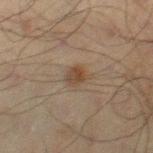The lesion was tiled from a total-body skin photograph and was not biopsied. The total-body-photography lesion software estimated a lesion area of about 4 mm² and two-axis asymmetry of about 0.3. It also reported a mean CIELAB color near L≈36 a*≈13 b*≈24, roughly 7 lightness units darker than nearby skin, and a normalized border contrast of about 7.5. The analysis additionally found a border-irregularity rating of about 3/10, a color-variation rating of about 4/10, and a peripheral color-asymmetry measure near 1.5. It also reported a detector confidence of about 100 out of 100 that the crop contains a lesion. A male subject about 70 years old. The lesion's longest dimension is about 3 mm. This is a cross-polarized tile. A 15 mm close-up extracted from a 3D total-body photography capture. The lesion is on the right thigh.